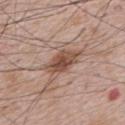Imaged during a routine full-body skin examination; the lesion was not biopsied and no histopathology is available. A male subject aged around 65. Cropped from a total-body skin-imaging series; the visible field is about 15 mm. Measured at roughly 6 mm in maximum diameter. Imaged with white-light lighting. The lesion is on the upper back.